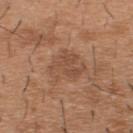{"patient": {"sex": "male", "age_approx": 40}, "site": "upper back", "image": {"source": "total-body photography crop", "field_of_view_mm": 15}, "lesion_size": {"long_diameter_mm_approx": 4.0}}